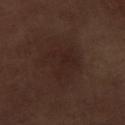follow-up: catalogued during a skin exam; not biopsied
subject: male, about 70 years old
automated metrics: a footprint of about 16 mm² and two-axis asymmetry of about 0.35; a mean CIELAB color near L≈23 a*≈15 b*≈19, a lesion–skin lightness drop of about 4, and a lesion-to-skin contrast of about 5 (normalized; higher = more distinct); border irregularity of about 4 on a 0–10 scale, a within-lesion color-variation index near 2.5/10, and peripheral color asymmetry of about 1; a classifier nevus-likeness of about 10/100 and a detector confidence of about 100 out of 100 that the crop contains a lesion
body site: the right lower leg
illumination: white-light
imaging modality: total-body-photography crop, ~15 mm field of view
lesion diameter: ~5 mm (longest diameter)